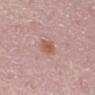Assessment: Imaged during a routine full-body skin examination; the lesion was not biopsied and no histopathology is available. Clinical summary: The recorded lesion diameter is about 2.5 mm. A male subject, roughly 50 years of age. Cropped from a total-body skin-imaging series; the visible field is about 15 mm. Captured under white-light illumination. The lesion is located on the right lower leg.